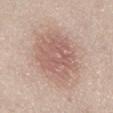follow-up: no biopsy performed (imaged during a skin exam); anatomic site: the lower back; illumination: white-light; diameter: about 7.5 mm; patient: male, in their mid- to late 70s; imaging modality: ~15 mm crop, total-body skin-cancer survey.